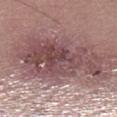Part of a total-body skin-imaging series; this lesion was reviewed on a skin check and was not flagged for biopsy.
A 15 mm crop from a total-body photograph taken for skin-cancer surveillance.
About 10.5 mm across.
Automated tile analysis of the lesion measured internal color variation of about 6 on a 0–10 scale and peripheral color asymmetry of about 2.
From the left lower leg.
The subject is a male aged approximately 35.
This is a white-light tile.